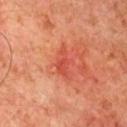Imaged during a routine full-body skin examination; the lesion was not biopsied and no histopathology is available.
Captured under cross-polarized illumination.
A close-up tile cropped from a whole-body skin photograph, about 15 mm across.
Automated image analysis of the tile measured internal color variation of about 1.5 on a 0–10 scale and radial color variation of about 0.5. The software also gave a classifier nevus-likeness of about 5/100.
From the chest.
A male subject aged approximately 65.